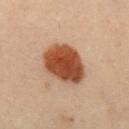Recorded during total-body skin imaging; not selected for excision or biopsy. A female subject, aged 28 to 32. The tile uses cross-polarized illumination. From the mid back. A region of skin cropped from a whole-body photographic capture, roughly 15 mm wide. The total-body-photography lesion software estimated a lesion-detection confidence of about 100/100. About 6.5 mm across.